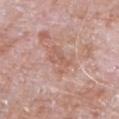<lesion>
  <biopsy_status>not biopsied; imaged during a skin examination</biopsy_status>
  <patient>
    <sex>male</sex>
    <age_approx>65</age_approx>
  </patient>
  <automated_metrics>
    <cielab_L>59</cielab_L>
    <cielab_a>21</cielab_a>
    <cielab_b>27</cielab_b>
    <vs_skin_darker_L>7.0</vs_skin_darker_L>
    <vs_skin_contrast_norm>5.5</vs_skin_contrast_norm>
    <nevus_likeness_0_100>0</nevus_likeness_0_100>
    <lesion_detection_confidence_0_100>95</lesion_detection_confidence_0_100>
  </automated_metrics>
  <image>
    <source>total-body photography crop</source>
    <field_of_view_mm>15</field_of_view_mm>
  </image>
  <site>chest</site>
  <lighting>white-light</lighting>
</lesion>